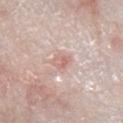Clinical impression: Part of a total-body skin-imaging series; this lesion was reviewed on a skin check and was not flagged for biopsy. Acquisition and patient details: The total-body-photography lesion software estimated an average lesion color of about L≈65 a*≈21 b*≈24 (CIELAB), about 9 CIELAB-L* units darker than the surrounding skin, and a normalized border contrast of about 5.5. It also reported border irregularity of about 2 on a 0–10 scale and radial color variation of about 1.5. It also reported a classifier nevus-likeness of about 0/100 and a detector confidence of about 90 out of 100 that the crop contains a lesion. A 15 mm close-up tile from a total-body photography series done for melanoma screening. The subject is a male approximately 65 years of age. Located on the right lower leg. Measured at roughly 2.5 mm in maximum diameter.